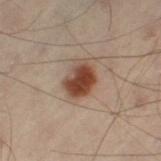{
  "site": "left thigh",
  "lesion_size": {
    "long_diameter_mm_approx": 4.0
  },
  "patient": {
    "sex": "male",
    "age_approx": 50
  },
  "automated_metrics": {
    "nevus_likeness_0_100": 100,
    "lesion_detection_confidence_0_100": 100
  },
  "image": {
    "source": "total-body photography crop",
    "field_of_view_mm": 15
  }
}